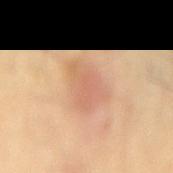{
  "biopsy_status": "not biopsied; imaged during a skin examination",
  "site": "back",
  "image": {
    "source": "total-body photography crop",
    "field_of_view_mm": 15
  },
  "lesion_size": {
    "long_diameter_mm_approx": 5.0
  },
  "lighting": "cross-polarized",
  "automated_metrics": {
    "area_mm2_approx": 12.0,
    "eccentricity": 0.7,
    "shape_asymmetry": 0.3,
    "cielab_L": 65,
    "cielab_a": 22,
    "cielab_b": 34,
    "vs_skin_darker_L": 8.0,
    "vs_skin_contrast_norm": 5.0,
    "nevus_likeness_0_100": 5,
    "lesion_detection_confidence_0_100": 100
  },
  "patient": {
    "sex": "male",
    "age_approx": 55
  }
}